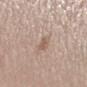Part of a total-body skin-imaging series; this lesion was reviewed on a skin check and was not flagged for biopsy.
A 15 mm crop from a total-body photograph taken for skin-cancer surveillance.
The patient is a male roughly 60 years of age.
From the left forearm.
The tile uses white-light illumination.